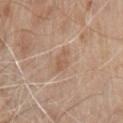Q: Was a biopsy performed?
A: catalogued during a skin exam; not biopsied
Q: What is the imaging modality?
A: ~15 mm tile from a whole-body skin photo
Q: Who is the patient?
A: male, aged around 80
Q: Lesion location?
A: the chest